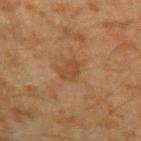This lesion was catalogued during total-body skin photography and was not selected for biopsy. From the left upper arm. An algorithmic analysis of the crop reported an eccentricity of roughly 0.8 and two-axis asymmetry of about 0.3. The software also gave a nevus-likeness score of about 0/100 and lesion-presence confidence of about 100/100. This is a cross-polarized tile. A male patient about 45 years old. Cropped from a total-body skin-imaging series; the visible field is about 15 mm.